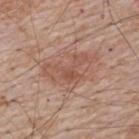follow-up = total-body-photography surveillance lesion; no biopsy
patient = male, aged approximately 65
site = the upper back
acquisition = ~15 mm tile from a whole-body skin photo